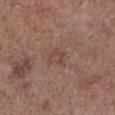The subject is a male roughly 60 years of age.
Located on the left lower leg.
A close-up tile cropped from a whole-body skin photograph, about 15 mm across.
The lesion's longest dimension is about 3 mm.